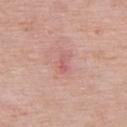No biopsy was performed on this lesion — it was imaged during a full skin examination and was not determined to be concerning. The subject is a male approximately 65 years of age. On the back. A region of skin cropped from a whole-body photographic capture, roughly 15 mm wide.